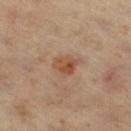<lesion>
<biopsy_status>not biopsied; imaged during a skin examination</biopsy_status>
<lighting>cross-polarized</lighting>
<lesion_size>
  <long_diameter_mm_approx>3.0</long_diameter_mm_approx>
</lesion_size>
<patient>
  <sex>male</sex>
  <age_approx>60</age_approx>
</patient>
<image>
  <source>total-body photography crop</source>
  <field_of_view_mm>15</field_of_view_mm>
</image>
<automated_metrics>
  <cielab_L>46</cielab_L>
  <cielab_a>19</cielab_a>
  <cielab_b>31</cielab_b>
  <vs_skin_darker_L>8.0</vs_skin_darker_L>
  <vs_skin_contrast_norm>7.5</vs_skin_contrast_norm>
  <border_irregularity_0_10>2.5</border_irregularity_0_10>
  <color_variation_0_10>5.0</color_variation_0_10>
</automated_metrics>
<site>right thigh</site>
</lesion>